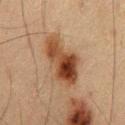Q: Was a biopsy performed?
A: imaged on a skin check; not biopsied
Q: What are the patient's age and sex?
A: male, aged around 60
Q: Lesion size?
A: ~7 mm (longest diameter)
Q: Illumination type?
A: cross-polarized
Q: How was this image acquired?
A: total-body-photography crop, ~15 mm field of view
Q: What is the anatomic site?
A: the abdomen